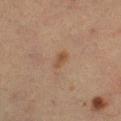notes: no biopsy performed (imaged during a skin exam)
diameter: ~2.5 mm (longest diameter)
anatomic site: the right lower leg
tile lighting: cross-polarized illumination
patient: female, approximately 55 years of age
image source: ~15 mm tile from a whole-body skin photo